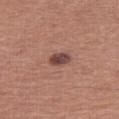<lesion>
<biopsy_status>not biopsied; imaged during a skin examination</biopsy_status>
<image>
  <source>total-body photography crop</source>
  <field_of_view_mm>15</field_of_view_mm>
</image>
<lesion_size>
  <long_diameter_mm_approx>2.5</long_diameter_mm_approx>
</lesion_size>
<patient>
  <sex>female</sex>
  <age_approx>55</age_approx>
</patient>
<automated_metrics>
  <nevus_likeness_0_100>70</nevus_likeness_0_100>
</automated_metrics>
<site>right thigh</site>
<lighting>white-light</lighting>
</lesion>